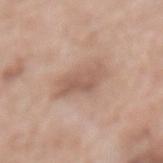Acquisition and patient details: A 15 mm close-up extracted from a 3D total-body photography capture. Captured under white-light illumination. The lesion-visualizer software estimated an outline eccentricity of about 0.85 (0 = round, 1 = elongated) and a symmetry-axis asymmetry near 0.35. And it measured a lesion–skin lightness drop of about 9 and a normalized border contrast of about 6. A female patient, in their mid-70s. The lesion is located on the mid back.